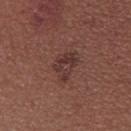follow-up — total-body-photography surveillance lesion; no biopsy
subject — female, aged 23–27
imaging modality — ~15 mm tile from a whole-body skin photo
anatomic site — the upper back